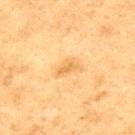No biopsy was performed on this lesion — it was imaged during a full skin examination and was not determined to be concerning.